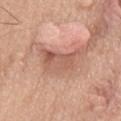This lesion was catalogued during total-body skin photography and was not selected for biopsy. The lesion is on the chest. Automated image analysis of the tile measured a nevus-likeness score of about 0/100 and a detector confidence of about 85 out of 100 that the crop contains a lesion. A male patient, aged 73–77. The lesion's longest dimension is about 6 mm. The tile uses white-light illumination. Cropped from a whole-body photographic skin survey; the tile spans about 15 mm.